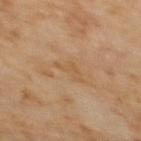subject: female, approximately 70 years of age | diameter: ~3.5 mm (longest diameter) | tile lighting: cross-polarized | anatomic site: the upper back | image: ~15 mm tile from a whole-body skin photo | image-analysis metrics: an average lesion color of about L≈47 a*≈15 b*≈32 (CIELAB), roughly 4 lightness units darker than nearby skin, and a lesion-to-skin contrast of about 4.5 (normalized; higher = more distinct); a border-irregularity rating of about 6.5/10, a color-variation rating of about 0.5/10, and radial color variation of about 0; a classifier nevus-likeness of about 0/100 and lesion-presence confidence of about 100/100.